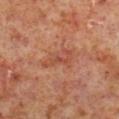Notes:
* notes · imaged on a skin check; not biopsied
* lesion size · ≈4 mm
* image · ~15 mm tile from a whole-body skin photo
* subject · male, aged around 60
* automated metrics · a lesion area of about 5 mm² and a symmetry-axis asymmetry near 0.5; a lesion color around L≈46 a*≈26 b*≈30 in CIELAB, a lesion–skin lightness drop of about 7, and a normalized border contrast of about 5.5
* body site · the right lower leg